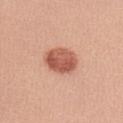Acquisition and patient details:
Located on the left forearm. A female patient about 25 years old. A region of skin cropped from a whole-body photographic capture, roughly 15 mm wide. Captured under white-light illumination. About 4 mm across.